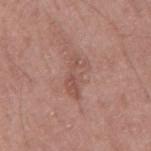  biopsy_status: not biopsied; imaged during a skin examination
  lesion_size:
    long_diameter_mm_approx: 5.0
  patient:
    sex: male
    age_approx: 60
  image:
    source: total-body photography crop
    field_of_view_mm: 15
  lighting: white-light
  site: mid back